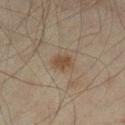The lesion was photographed on a routine skin check and not biopsied; there is no pathology result. The patient is a male aged 43–47. Captured under cross-polarized illumination. A region of skin cropped from a whole-body photographic capture, roughly 15 mm wide. From the left lower leg.